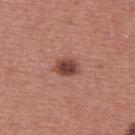Imaged during a routine full-body skin examination; the lesion was not biopsied and no histopathology is available.
Cropped from a total-body skin-imaging series; the visible field is about 15 mm.
The total-body-photography lesion software estimated a within-lesion color-variation index near 3.5/10.
A male subject aged around 40.
Captured under white-light illumination.
Longest diameter approximately 3 mm.
Located on the upper back.